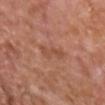The lesion's longest dimension is about 4 mm.
A 15 mm close-up extracted from a 3D total-body photography capture.
The lesion is on the chest.
A male subject, in their mid- to late 60s.
The tile uses white-light illumination.